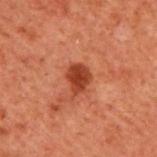Captured during whole-body skin photography for melanoma surveillance; the lesion was not biopsied.
Approximately 3 mm at its widest.
A male patient, about 60 years old.
On the back.
A 15 mm close-up extracted from a 3D total-body photography capture.
The lesion-visualizer software estimated a lesion area of about 6 mm², an eccentricity of roughly 0.65, and a shape-asymmetry score of about 0.25 (0 = symmetric). The analysis additionally found a classifier nevus-likeness of about 80/100.
Captured under cross-polarized illumination.